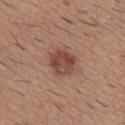workup: total-body-photography surveillance lesion; no biopsy
automated metrics: a lesion area of about 8.5 mm², an eccentricity of roughly 0.5, and a shape-asymmetry score of about 0.2 (0 = symmetric); a classifier nevus-likeness of about 90/100 and a lesion-detection confidence of about 100/100
site: the mid back
subject: male, about 60 years old
image: 15 mm crop, total-body photography
lighting: white-light illumination